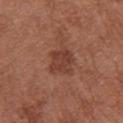body site=the left arm | tile lighting=white-light illumination | image source=total-body-photography crop, ~15 mm field of view | diameter=~3.5 mm (longest diameter) | subject=female, in their mid-60s.